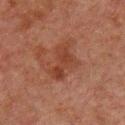{
  "biopsy_status": "not biopsied; imaged during a skin examination",
  "image": {
    "source": "total-body photography crop",
    "field_of_view_mm": 15
  },
  "automated_metrics": {
    "area_mm2_approx": 8.0,
    "eccentricity": 0.8
  },
  "site": "chest",
  "lighting": "cross-polarized",
  "patient": {
    "sex": "male",
    "age_approx": 60
  }
}